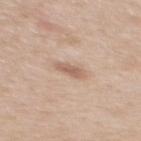Notes:
– workup · catalogued during a skin exam; not biopsied
– image · ~15 mm tile from a whole-body skin photo
– image-analysis metrics · a lesion area of about 4 mm², an eccentricity of roughly 0.9, and two-axis asymmetry of about 0.2; a normalized border contrast of about 6.5
– lesion size · about 3 mm
– location · the upper back
– patient · female, aged approximately 40